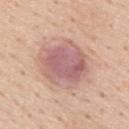Findings:
- notes — no biopsy performed (imaged during a skin exam)
- image-analysis metrics — two-axis asymmetry of about 0.15; a mean CIELAB color near L≈61 a*≈22 b*≈22, about 11 CIELAB-L* units darker than the surrounding skin, and a normalized border contrast of about 8.5; a border-irregularity index near 2/10 and a within-lesion color-variation index near 5.5/10; an automated nevus-likeness rating near 5 out of 100 and a detector confidence of about 100 out of 100 that the crop contains a lesion
- image — ~15 mm tile from a whole-body skin photo
- patient — male, approximately 60 years of age
- lesion size — about 6 mm
- location — the upper back